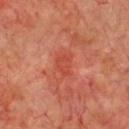Clinical impression:
The lesion was photographed on a routine skin check and not biopsied; there is no pathology result.
Background:
Captured under cross-polarized illumination. The lesion is located on the chest. A 15 mm close-up extracted from a 3D total-body photography capture. A male subject, approximately 70 years of age. The recorded lesion diameter is about 3 mm.